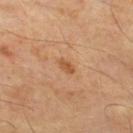Captured during whole-body skin photography for melanoma surveillance; the lesion was not biopsied. The lesion is on the upper back. A male subject, approximately 55 years of age. Cropped from a total-body skin-imaging series; the visible field is about 15 mm. The lesion-visualizer software estimated a lesion area of about 2.5 mm², an eccentricity of roughly 0.85, and two-axis asymmetry of about 0.25. The analysis additionally found a border-irregularity index near 2/10. It also reported a classifier nevus-likeness of about 45/100 and lesion-presence confidence of about 100/100. This is a cross-polarized tile.